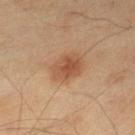Impression: Imaged during a routine full-body skin examination; the lesion was not biopsied and no histopathology is available. Background: Approximately 4 mm at its widest. The lesion is on the left lower leg. A male subject, roughly 70 years of age. A roughly 15 mm field-of-view crop from a total-body skin photograph. This is a cross-polarized tile.